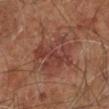Assessment: Part of a total-body skin-imaging series; this lesion was reviewed on a skin check and was not flagged for biopsy. Image and clinical context: A male subject in their 60s. Located on the right leg. A roughly 15 mm field-of-view crop from a total-body skin photograph.